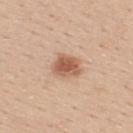This lesion was catalogued during total-body skin photography and was not selected for biopsy. A lesion tile, about 15 mm wide, cut from a 3D total-body photograph. Located on the upper back. The patient is a male roughly 30 years of age.